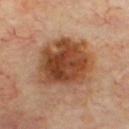Q: Was this lesion biopsied?
A: total-body-photography surveillance lesion; no biopsy
Q: Where on the body is the lesion?
A: the chest
Q: Patient demographics?
A: male, in their 70s
Q: How was this image acquired?
A: ~15 mm tile from a whole-body skin photo